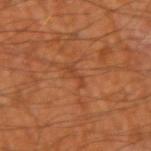Case summary:
* biopsy status: total-body-photography surveillance lesion; no biopsy
* automated metrics: a lesion area of about 2 mm², a shape eccentricity near 0.95, and a symmetry-axis asymmetry near 0.5; a classifier nevus-likeness of about 0/100 and a detector confidence of about 100 out of 100 that the crop contains a lesion
* subject: male, about 60 years old
* size: ≈2.5 mm
* imaging modality: total-body-photography crop, ~15 mm field of view
* tile lighting: cross-polarized
* location: the right upper arm